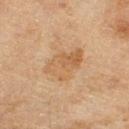Acquisition and patient details:
The tile uses cross-polarized illumination. The lesion is located on the right thigh. Automated image analysis of the tile measured an automated nevus-likeness rating near 5 out of 100 and lesion-presence confidence of about 100/100. The lesion's longest dimension is about 5 mm. This image is a 15 mm lesion crop taken from a total-body photograph. A female patient, approximately 60 years of age.